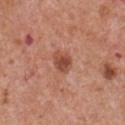Impression: Recorded during total-body skin imaging; not selected for excision or biopsy. Background: The subject is a male roughly 65 years of age. On the chest. Automated tile analysis of the lesion measured a border-irregularity rating of about 2/10, a within-lesion color-variation index near 3.5/10, and peripheral color asymmetry of about 1. It also reported a nevus-likeness score of about 90/100 and lesion-presence confidence of about 100/100. A close-up tile cropped from a whole-body skin photograph, about 15 mm across. The lesion's longest dimension is about 3 mm.